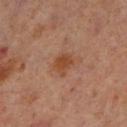size — ~2.5 mm (longest diameter)
image — ~15 mm tile from a whole-body skin photo
tile lighting — cross-polarized
patient — male, aged approximately 60
TBP lesion metrics — an area of roughly 5 mm², an eccentricity of roughly 0.65, and two-axis asymmetry of about 0.25; a mean CIELAB color near L≈42 a*≈22 b*≈32 and about 8 CIELAB-L* units darker than the surrounding skin; border irregularity of about 2.5 on a 0–10 scale, internal color variation of about 2 on a 0–10 scale, and peripheral color asymmetry of about 0.5; an automated nevus-likeness rating near 30 out of 100 and a lesion-detection confidence of about 100/100
anatomic site — the left lower leg